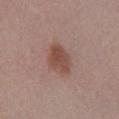The lesion was tiled from a total-body skin photograph and was not biopsied. This is a white-light tile. The subject is a female aged approximately 40. A roughly 15 mm field-of-view crop from a total-body skin photograph. The lesion's longest dimension is about 4 mm. From the right lower leg.